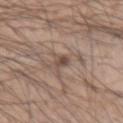This lesion was catalogued during total-body skin photography and was not selected for biopsy.
A male subject in their mid-30s.
A region of skin cropped from a whole-body photographic capture, roughly 15 mm wide.
Captured under white-light illumination.
Automated image analysis of the tile measured a lesion color around L≈48 a*≈15 b*≈24 in CIELAB, about 10 CIELAB-L* units darker than the surrounding skin, and a lesion-to-skin contrast of about 7 (normalized; higher = more distinct). The analysis additionally found a border-irregularity rating of about 3.5/10 and internal color variation of about 3.5 on a 0–10 scale. It also reported a classifier nevus-likeness of about 30/100 and lesion-presence confidence of about 100/100.
On the arm.